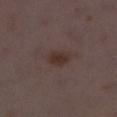This lesion was catalogued during total-body skin photography and was not selected for biopsy.
A 15 mm crop from a total-body photograph taken for skin-cancer surveillance.
The recorded lesion diameter is about 3 mm.
The lesion is on the right lower leg.
A female patient, in their 30s.
Captured under white-light illumination.